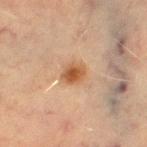Captured during whole-body skin photography for melanoma surveillance; the lesion was not biopsied.
The patient is a male aged 68 to 72.
On the right thigh.
Cropped from a whole-body photographic skin survey; the tile spans about 15 mm.